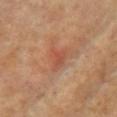follow-up: total-body-photography surveillance lesion; no biopsy | location: the upper back | illumination: cross-polarized | lesion diameter: about 3 mm | TBP lesion metrics: a footprint of about 5 mm², an outline eccentricity of about 0.7 (0 = round, 1 = elongated), and a shape-asymmetry score of about 0.45 (0 = symmetric); internal color variation of about 2 on a 0–10 scale and peripheral color asymmetry of about 0.5; a nevus-likeness score of about 0/100 and lesion-presence confidence of about 95/100 | image: ~15 mm crop, total-body skin-cancer survey | subject: female, in their mid- to late 70s.